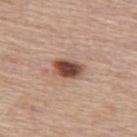  patient:
    sex: female
    age_approx: 65
  image:
    source: total-body photography crop
    field_of_view_mm: 15
  site: back